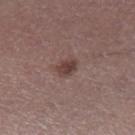The total-body-photography lesion software estimated a footprint of about 4 mm², an outline eccentricity of about 0.7 (0 = round, 1 = elongated), and two-axis asymmetry of about 0.3. The analysis additionally found a lesion color around L≈40 a*≈18 b*≈20 in CIELAB and a normalized border contrast of about 8.5. Imaged with white-light lighting. Longest diameter approximately 2.5 mm. A close-up tile cropped from a whole-body skin photograph, about 15 mm across. From the left lower leg. The subject is a male aged around 55.